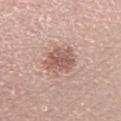Captured during whole-body skin photography for melanoma surveillance; the lesion was not biopsied. A male patient aged around 65. This is a white-light tile. A 15 mm close-up tile from a total-body photography series done for melanoma screening. About 4 mm across. The lesion is on the left lower leg.